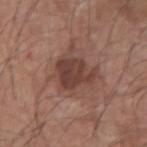Assessment:
Captured during whole-body skin photography for melanoma surveillance; the lesion was not biopsied.
Image and clinical context:
A male patient aged 68 to 72. This is a white-light tile. The lesion's longest dimension is about 4.5 mm. A lesion tile, about 15 mm wide, cut from a 3D total-body photograph. From the left forearm.